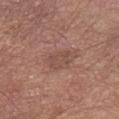* TBP lesion metrics: a lesion area of about 4.5 mm², an eccentricity of roughly 0.75, and two-axis asymmetry of about 0.4; a mean CIELAB color near L≈48 a*≈20 b*≈24; internal color variation of about 1.5 on a 0–10 scale and radial color variation of about 0.5
* subject: male, aged 73 to 77
* illumination: white-light illumination
* lesion size: about 3 mm
* acquisition: ~15 mm tile from a whole-body skin photo
* location: the left forearm